A male patient about 75 years old. A region of skin cropped from a whole-body photographic capture, roughly 15 mm wide. The tile uses white-light illumination. On the back:
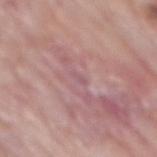Diagnosis: On excision, pathology confirmed a nodular basal cell carcinoma.